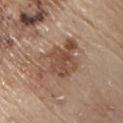{
  "biopsy_status": "not biopsied; imaged during a skin examination",
  "image": {
    "source": "total-body photography crop",
    "field_of_view_mm": 15
  },
  "site": "chest",
  "lesion_size": {
    "long_diameter_mm_approx": 6.0
  },
  "automated_metrics": {
    "cielab_L": 49,
    "cielab_a": 19,
    "cielab_b": 28,
    "vs_skin_darker_L": 10.0,
    "vs_skin_contrast_norm": 7.5,
    "border_irregularity_0_10": 7.0,
    "color_variation_0_10": 4.0,
    "peripheral_color_asymmetry": 1.0,
    "nevus_likeness_0_100": 5,
    "lesion_detection_confidence_0_100": 100
  },
  "patient": {
    "sex": "male",
    "age_approx": 80
  },
  "lighting": "white-light"
}